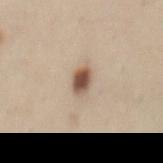Part of a total-body skin-imaging series; this lesion was reviewed on a skin check and was not flagged for biopsy.
Located on the back.
This image is a 15 mm lesion crop taken from a total-body photograph.
The patient is a female aged 38–42.
This is a white-light tile.
An algorithmic analysis of the crop reported a color-variation rating of about 5/10 and radial color variation of about 1. And it measured a classifier nevus-likeness of about 100/100 and a detector confidence of about 100 out of 100 that the crop contains a lesion.